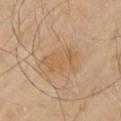Assessment:
Imaged during a routine full-body skin examination; the lesion was not biopsied and no histopathology is available.
Background:
The lesion is on the left upper arm. A male patient, aged around 65. A region of skin cropped from a whole-body photographic capture, roughly 15 mm wide. About 5 mm across.